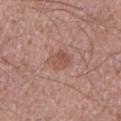Imaged during a routine full-body skin examination; the lesion was not biopsied and no histopathology is available. This is a white-light tile. A 15 mm crop from a total-body photograph taken for skin-cancer surveillance. The subject is a male aged approximately 35. Approximately 2.5 mm at its widest. From the right upper arm.